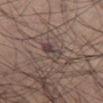biopsy status: catalogued during a skin exam; not biopsied
patient: male, roughly 55 years of age
image-analysis metrics: a symmetry-axis asymmetry near 0.3; a border-irregularity index near 3/10, a color-variation rating of about 9/10, and radial color variation of about 3; an automated nevus-likeness rating near 0 out of 100 and lesion-presence confidence of about 50/100
illumination: white-light illumination
lesion diameter: ~3 mm (longest diameter)
acquisition: ~15 mm crop, total-body skin-cancer survey
location: the left thigh